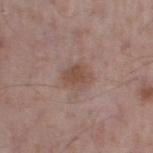Recorded during total-body skin imaging; not selected for excision or biopsy. The lesion is located on the right lower leg. The patient is a male aged around 55. A 15 mm crop from a total-body photograph taken for skin-cancer surveillance. The tile uses white-light illumination.